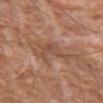Findings:
• workup: no biopsy performed (imaged during a skin exam)
• patient: male, about 75 years old
• anatomic site: the left upper arm
• image source: ~15 mm crop, total-body skin-cancer survey